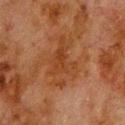No biopsy was performed on this lesion — it was imaged during a full skin examination and was not determined to be concerning.
The lesion is on the back.
The patient is a male aged 78 to 82.
About 6 mm across.
This is a cross-polarized tile.
A 15 mm crop from a total-body photograph taken for skin-cancer surveillance.